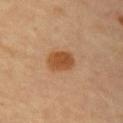No biopsy was performed on this lesion — it was imaged during a full skin examination and was not determined to be concerning. The patient is aged approximately 60. On the right upper arm. A 15 mm close-up tile from a total-body photography series done for melanoma screening.